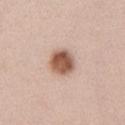biopsy status: no biopsy performed (imaged during a skin exam); subject: female, aged approximately 40; image source: total-body-photography crop, ~15 mm field of view; location: the left upper arm; image-analysis metrics: a lesion area of about 8 mm², an outline eccentricity of about 0.4 (0 = round, 1 = elongated), and a symmetry-axis asymmetry near 0.15.